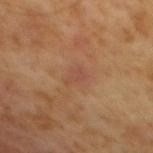notes: catalogued during a skin exam; not biopsied | image source: ~15 mm tile from a whole-body skin photo | subject: male, aged 58 to 62 | lesion size: about 2.5 mm | body site: the back | automated lesion analysis: an area of roughly 3 mm², an eccentricity of roughly 0.8, and a symmetry-axis asymmetry near 0.4; a mean CIELAB color near L≈43 a*≈21 b*≈28, about 5 CIELAB-L* units darker than the surrounding skin, and a normalized lesion–skin contrast near 4.5; border irregularity of about 4 on a 0–10 scale, a color-variation rating of about 1.5/10, and peripheral color asymmetry of about 0.5; lesion-presence confidence of about 100/100.